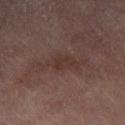| field | value |
|---|---|
| tile lighting | cross-polarized |
| patient | female, aged around 80 |
| image | 15 mm crop, total-body photography |
| anatomic site | the right leg |
| lesion size | about 5.5 mm |